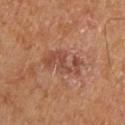The lesion was tiled from a total-body skin photograph and was not biopsied. The subject is a male approximately 65 years of age. From the left upper arm. About 4.5 mm across. Cropped from a whole-body photographic skin survey; the tile spans about 15 mm. Imaged with cross-polarized lighting.